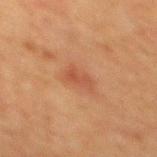Recorded during total-body skin imaging; not selected for excision or biopsy. A roughly 15 mm field-of-view crop from a total-body skin photograph. The lesion is on the mid back. Longest diameter approximately 3 mm. A male subject, aged 48 to 52. This is a cross-polarized tile. The lesion-visualizer software estimated a lesion area of about 3 mm², a shape eccentricity near 0.9, and a symmetry-axis asymmetry near 0.35. And it measured a border-irregularity rating of about 4.5/10.